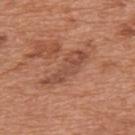Part of a total-body skin-imaging series; this lesion was reviewed on a skin check and was not flagged for biopsy. A roughly 15 mm field-of-view crop from a total-body skin photograph. Automated tile analysis of the lesion measured a lesion area of about 8.5 mm² and two-axis asymmetry of about 0.45. The analysis additionally found a color-variation rating of about 3/10 and radial color variation of about 1. The analysis additionally found a detector confidence of about 95 out of 100 that the crop contains a lesion. This is a white-light tile. The subject is a male aged around 65. From the mid back. About 6 mm across.